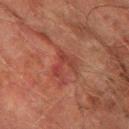Imaged during a routine full-body skin examination; the lesion was not biopsied and no histopathology is available. A 15 mm close-up extracted from a 3D total-body photography capture. The subject is a male in their mid- to late 70s. Longest diameter approximately 3.5 mm. Located on the left thigh. The lesion-visualizer software estimated an area of roughly 5.5 mm², an eccentricity of roughly 0.65, and a shape-asymmetry score of about 0.7 (0 = symmetric). And it measured a mean CIELAB color near L≈35 a*≈25 b*≈25, about 6 CIELAB-L* units darker than the surrounding skin, and a normalized border contrast of about 6.